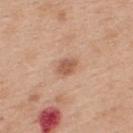Q: Was a biopsy performed?
A: imaged on a skin check; not biopsied
Q: Illumination type?
A: white-light illumination
Q: Where on the body is the lesion?
A: the upper back
Q: What is the imaging modality?
A: ~15 mm crop, total-body skin-cancer survey
Q: What are the patient's age and sex?
A: female, aged 38–42